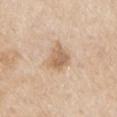Q: Is there a histopathology result?
A: total-body-photography surveillance lesion; no biopsy
Q: What is the lesion's diameter?
A: ~3.5 mm (longest diameter)
Q: How was the tile lit?
A: white-light
Q: Lesion location?
A: the left upper arm
Q: What kind of image is this?
A: ~15 mm tile from a whole-body skin photo
Q: Patient demographics?
A: male, approximately 80 years of age
Q: Automated lesion metrics?
A: a footprint of about 7 mm², an eccentricity of roughly 0.55, and a shape-asymmetry score of about 0.3 (0 = symmetric); an average lesion color of about L≈63 a*≈17 b*≈33 (CIELAB), a lesion–skin lightness drop of about 11, and a normalized lesion–skin contrast near 7